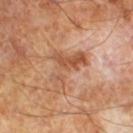{
  "biopsy_status": "not biopsied; imaged during a skin examination",
  "image": {
    "source": "total-body photography crop",
    "field_of_view_mm": 15
  },
  "patient": {
    "sex": "male",
    "age_approx": 65
  }
}